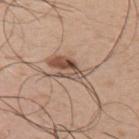Impression:
This lesion was catalogued during total-body skin photography and was not selected for biopsy.
Clinical summary:
Imaged with white-light lighting. Approximately 6 mm at its widest. The lesion is on the upper back. A roughly 15 mm field-of-view crop from a total-body skin photograph. A male subject approximately 50 years of age. The lesion-visualizer software estimated a lesion area of about 10 mm² and two-axis asymmetry of about 0.45. And it measured an average lesion color of about L≈53 a*≈17 b*≈27 (CIELAB), a lesion–skin lightness drop of about 12, and a normalized lesion–skin contrast near 8. And it measured a classifier nevus-likeness of about 45/100 and a detector confidence of about 60 out of 100 that the crop contains a lesion.